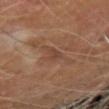Q: Is there a histopathology result?
A: imaged on a skin check; not biopsied
Q: How large is the lesion?
A: ~2.5 mm (longest diameter)
Q: What did automated image analysis measure?
A: a footprint of about 3 mm² and an outline eccentricity of about 0.85 (0 = round, 1 = elongated); a mean CIELAB color near L≈41 a*≈19 b*≈27, about 7 CIELAB-L* units darker than the surrounding skin, and a normalized lesion–skin contrast near 5.5
Q: Who is the patient?
A: male, in their 70s
Q: Lesion location?
A: the right forearm
Q: What lighting was used for the tile?
A: cross-polarized
Q: How was this image acquired?
A: ~15 mm crop, total-body skin-cancer survey